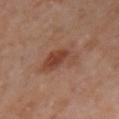<case>
  <patient>
    <sex>female</sex>
    <age_approx>50</age_approx>
  </patient>
  <automated_metrics>
    <cielab_L>42</cielab_L>
    <cielab_a>21</cielab_a>
    <cielab_b>27</cielab_b>
    <vs_skin_darker_L>8.0</vs_skin_darker_L>
    <vs_skin_contrast_norm>7.0</vs_skin_contrast_norm>
    <color_variation_0_10>7.0</color_variation_0_10>
    <peripheral_color_asymmetry>2.0</peripheral_color_asymmetry>
    <nevus_likeness_0_100>70</nevus_likeness_0_100>
    <lesion_detection_confidence_0_100>100</lesion_detection_confidence_0_100>
  </automated_metrics>
  <image>
    <source>total-body photography crop</source>
    <field_of_view_mm>15</field_of_view_mm>
  </image>
  <lesion_size>
    <long_diameter_mm_approx>5.0</long_diameter_mm_approx>
  </lesion_size>
  <site>right upper arm</site>
  <lighting>cross-polarized</lighting>
</case>